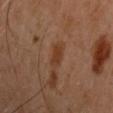workup = imaged on a skin check; not biopsied
patient = male, aged 43–47
anatomic site = the left upper arm
automated metrics = a lesion color around L≈34 a*≈20 b*≈30 in CIELAB and a normalized lesion–skin contrast near 8; a border-irregularity rating of about 3.5/10, a color-variation rating of about 1/10, and a peripheral color-asymmetry measure near 0.5
tile lighting = cross-polarized
acquisition = 15 mm crop, total-body photography